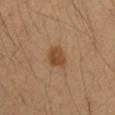acquisition = ~15 mm crop, total-body skin-cancer survey
body site = the right forearm
illumination = cross-polarized
lesion size = ~2.5 mm (longest diameter)
automated lesion analysis = a mean CIELAB color near L≈46 a*≈20 b*≈35 and a normalized lesion–skin contrast near 8; lesion-presence confidence of about 100/100
subject = female, aged around 35